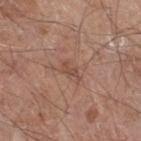Q: Is there a histopathology result?
A: imaged on a skin check; not biopsied